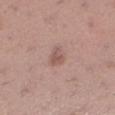Q: Was this lesion biopsied?
A: imaged on a skin check; not biopsied
Q: How large is the lesion?
A: ≈2.5 mm
Q: What is the anatomic site?
A: the left lower leg
Q: What is the imaging modality?
A: total-body-photography crop, ~15 mm field of view
Q: What lighting was used for the tile?
A: white-light
Q: Patient demographics?
A: female, aged 28 to 32
Q: What did automated image analysis measure?
A: an average lesion color of about L≈54 a*≈20 b*≈23 (CIELAB), roughly 9 lightness units darker than nearby skin, and a lesion-to-skin contrast of about 6 (normalized; higher = more distinct); a nevus-likeness score of about 20/100